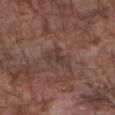workup = catalogued during a skin exam; not biopsied
patient = male, about 75 years old
tile lighting = white-light illumination
lesion diameter = about 3.5 mm
body site = the right forearm
imaging modality = 15 mm crop, total-body photography